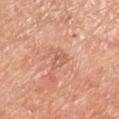  biopsy_status: not biopsied; imaged during a skin examination
  lesion_size:
    long_diameter_mm_approx: 3.0
  lighting: white-light
  image:
    source: total-body photography crop
    field_of_view_mm: 15
  site: right lower leg
  patient:
    sex: male
    age_approx: 80